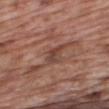notes: total-body-photography surveillance lesion; no biopsy
location: the upper back
subject: male, roughly 70 years of age
image: 15 mm crop, total-body photography